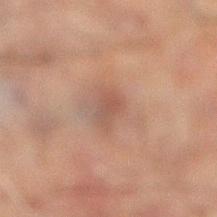The lesion was tiled from a total-body skin photograph and was not biopsied. A 15 mm close-up tile from a total-body photography series done for melanoma screening. The patient is a male roughly 60 years of age. Located on the left leg. The lesion's longest dimension is about 2.5 mm.